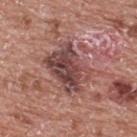  biopsy_status: not biopsied; imaged during a skin examination
  lighting: white-light
  automated_metrics:
    eccentricity: 0.7
    shape_asymmetry: 0.2
    border_irregularity_0_10: 3.0
    color_variation_0_10: 7.5
    peripheral_color_asymmetry: 2.5
  site: back
  patient:
    sex: male
    age_approx: 70
  image:
    source: total-body photography crop
    field_of_view_mm: 15
  lesion_size:
    long_diameter_mm_approx: 5.5